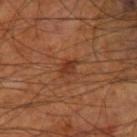biopsy status=no biopsy performed (imaged during a skin exam)
TBP lesion metrics=a border-irregularity index near 2.5/10 and internal color variation of about 2 on a 0–10 scale
subject=male, aged around 70
location=the right thigh
image source=~15 mm tile from a whole-body skin photo
size=about 2.5 mm